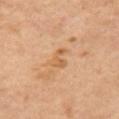Acquisition and patient details:
Imaged with cross-polarized lighting. The patient is a female roughly 45 years of age. Automated image analysis of the tile measured a lesion area of about 3.5 mm² and an outline eccentricity of about 0.8 (0 = round, 1 = elongated). It also reported a mean CIELAB color near L≈58 a*≈21 b*≈38, about 7 CIELAB-L* units darker than the surrounding skin, and a lesion-to-skin contrast of about 6 (normalized; higher = more distinct). Longest diameter approximately 2.5 mm. The lesion is located on the back. Cropped from a whole-body photographic skin survey; the tile spans about 15 mm.